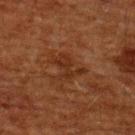Q: Is there a histopathology result?
A: imaged on a skin check; not biopsied
Q: What are the patient's age and sex?
A: male, aged 58 to 62
Q: Lesion location?
A: the upper back
Q: What lighting was used for the tile?
A: cross-polarized
Q: What is the imaging modality?
A: ~15 mm crop, total-body skin-cancer survey
Q: Lesion size?
A: about 3.5 mm
Q: What did automated image analysis measure?
A: a lesion area of about 5 mm², a shape eccentricity near 0.8, and a symmetry-axis asymmetry near 0.45; a mean CIELAB color near L≈23 a*≈19 b*≈25 and about 6 CIELAB-L* units darker than the surrounding skin; internal color variation of about 0.5 on a 0–10 scale and peripheral color asymmetry of about 0; a nevus-likeness score of about 0/100